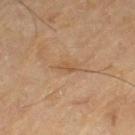biopsy status = total-body-photography surveillance lesion; no biopsy
image = 15 mm crop, total-body photography
subject = male, in their 70s
body site = the left thigh